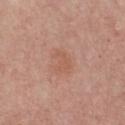Recorded during total-body skin imaging; not selected for excision or biopsy. The tile uses white-light illumination. Automated image analysis of the tile measured lesion-presence confidence of about 100/100. A 15 mm close-up extracted from a 3D total-body photography capture. A male patient aged around 60. Located on the chest. Measured at roughly 3.5 mm in maximum diameter.